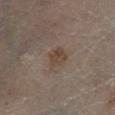| key | value |
|---|---|
| workup | catalogued during a skin exam; not biopsied |
| lesion size | ≈2.5 mm |
| automated metrics | an automated nevus-likeness rating near 0 out of 100 |
| body site | the right lower leg |
| tile lighting | cross-polarized illumination |
| subject | female, roughly 60 years of age |
| imaging modality | total-body-photography crop, ~15 mm field of view |